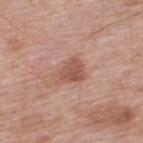The lesion was photographed on a routine skin check and not biopsied; there is no pathology result.
A male subject roughly 60 years of age.
The lesion is located on the upper back.
The lesion-visualizer software estimated a shape eccentricity near 0.7 and a symmetry-axis asymmetry near 0.35. The software also gave an average lesion color of about L≈54 a*≈23 b*≈28 (CIELAB), roughly 10 lightness units darker than nearby skin, and a lesion-to-skin contrast of about 7 (normalized; higher = more distinct). And it measured internal color variation of about 3 on a 0–10 scale and radial color variation of about 1. It also reported a classifier nevus-likeness of about 65/100 and lesion-presence confidence of about 100/100.
The lesion's longest dimension is about 4 mm.
Cropped from a whole-body photographic skin survey; the tile spans about 15 mm.
This is a white-light tile.